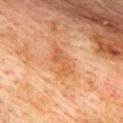This lesion was catalogued during total-body skin photography and was not selected for biopsy. A 15 mm crop from a total-body photograph taken for skin-cancer surveillance. The lesion is located on the upper back. A male subject roughly 75 years of age.